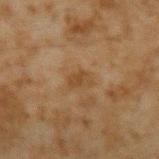workup — imaged on a skin check; not biopsied
patient — male, aged around 45
lighting — cross-polarized
anatomic site — the left upper arm
image — ~15 mm tile from a whole-body skin photo
lesion size — ≈2.5 mm